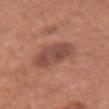{
  "biopsy_status": "not biopsied; imaged during a skin examination",
  "lesion_size": {
    "long_diameter_mm_approx": 5.0
  },
  "patient": {
    "sex": "female",
    "age_approx": 65
  },
  "site": "right forearm",
  "image": {
    "source": "total-body photography crop",
    "field_of_view_mm": 15
  },
  "automated_metrics": {
    "eccentricity": 0.65,
    "shape_asymmetry": 0.15,
    "cielab_L": 48,
    "cielab_a": 23,
    "cielab_b": 26,
    "vs_skin_darker_L": 10.0,
    "vs_skin_contrast_norm": 7.5,
    "color_variation_0_10": 4.0,
    "peripheral_color_asymmetry": 1.0,
    "nevus_likeness_0_100": 45,
    "lesion_detection_confidence_0_100": 100
  },
  "lighting": "white-light"
}